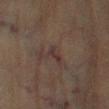• follow-up · total-body-photography surveillance lesion; no biopsy
• site · the right lower leg
• acquisition · total-body-photography crop, ~15 mm field of view
• subject · male, aged around 85 or older